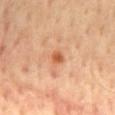Clinical impression: Recorded during total-body skin imaging; not selected for excision or biopsy. Acquisition and patient details: A male patient about 65 years old. A 15 mm crop from a total-body photograph taken for skin-cancer surveillance. Imaged with cross-polarized lighting. The lesion is on the mid back. The lesion's longest dimension is about 1.5 mm.